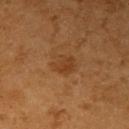Assessment: Captured during whole-body skin photography for melanoma surveillance; the lesion was not biopsied. Acquisition and patient details: On the right upper arm. A 15 mm close-up tile from a total-body photography series done for melanoma screening. A male patient, in their 60s.